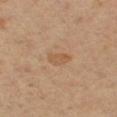Q: What are the patient's age and sex?
A: female, aged around 65
Q: How was the tile lit?
A: cross-polarized
Q: How was this image acquired?
A: 15 mm crop, total-body photography
Q: What did automated image analysis measure?
A: a lesion area of about 4 mm² and an eccentricity of roughly 0.6; a mean CIELAB color near L≈53 a*≈18 b*≈33, about 6 CIELAB-L* units darker than the surrounding skin, and a normalized lesion–skin contrast near 5.5; a classifier nevus-likeness of about 5/100 and a detector confidence of about 100 out of 100 that the crop contains a lesion
Q: Lesion location?
A: the left thigh
Q: What is the lesion's diameter?
A: ~2.5 mm (longest diameter)